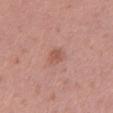* follow-up · total-body-photography surveillance lesion; no biopsy
* location · the left thigh
* imaging modality · 15 mm crop, total-body photography
* subject · female, aged 38–42
* illumination · white-light illumination
* lesion size · ≈2.5 mm